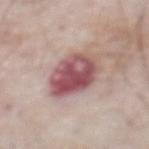image-analysis metrics: an automated nevus-likeness rating near 25 out of 100 and a detector confidence of about 100 out of 100 that the crop contains a lesion | location: the abdomen | imaging modality: ~15 mm crop, total-body skin-cancer survey | diameter: ~5.5 mm (longest diameter) | patient: male, aged around 75 | tile lighting: white-light illumination.